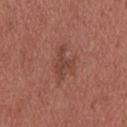follow-up=catalogued during a skin exam; not biopsied
body site=the upper back
image=~15 mm crop, total-body skin-cancer survey
patient=male, aged 23 to 27
tile lighting=white-light illumination